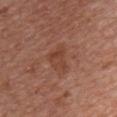Impression:
Imaged during a routine full-body skin examination; the lesion was not biopsied and no histopathology is available.
Acquisition and patient details:
A 15 mm close-up tile from a total-body photography series done for melanoma screening. Located on the chest. Automated image analysis of the tile measured border irregularity of about 4 on a 0–10 scale, internal color variation of about 3.5 on a 0–10 scale, and radial color variation of about 1. And it measured a nevus-likeness score of about 0/100 and a lesion-detection confidence of about 100/100. The lesion's longest dimension is about 4 mm. A male subject, aged around 65. Imaged with white-light lighting.